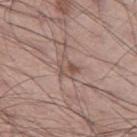The lesion was tiled from a total-body skin photograph and was not biopsied.
Imaged with white-light lighting.
Cropped from a total-body skin-imaging series; the visible field is about 15 mm.
A male patient aged around 45.
From the left thigh.